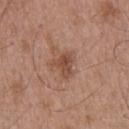| feature | finding |
|---|---|
| follow-up | total-body-photography surveillance lesion; no biopsy |
| acquisition | total-body-photography crop, ~15 mm field of view |
| TBP lesion metrics | a classifier nevus-likeness of about 10/100 and lesion-presence confidence of about 100/100 |
| subject | male, aged 48–52 |
| tile lighting | white-light |
| anatomic site | the upper back |